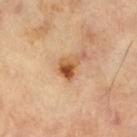Captured during whole-body skin photography for melanoma surveillance; the lesion was not biopsied. The subject is a male aged 63 to 67. The recorded lesion diameter is about 2.5 mm. A close-up tile cropped from a whole-body skin photograph, about 15 mm across. This is a cross-polarized tile.